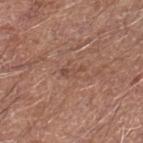Imaged during a routine full-body skin examination; the lesion was not biopsied and no histopathology is available. A 15 mm crop from a total-body photograph taken for skin-cancer surveillance. Captured under white-light illumination. The total-body-photography lesion software estimated an average lesion color of about L≈47 a*≈20 b*≈27 (CIELAB), roughly 7 lightness units darker than nearby skin, and a normalized lesion–skin contrast near 5. And it measured lesion-presence confidence of about 70/100. Approximately 3 mm at its widest. Located on the right lower leg. A male patient, aged 78–82.